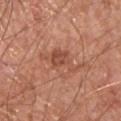Imaged during a routine full-body skin examination; the lesion was not biopsied and no histopathology is available. Located on the chest. This is a white-light tile. Cropped from a whole-body photographic skin survey; the tile spans about 15 mm. A male patient, in their mid- to late 60s. About 5.5 mm across.